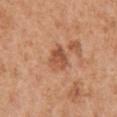Q: Was this lesion biopsied?
A: no biopsy performed (imaged during a skin exam)
Q: Where on the body is the lesion?
A: the arm
Q: Who is the patient?
A: female, aged around 40
Q: What is the lesion's diameter?
A: ~3 mm (longest diameter)
Q: How was the tile lit?
A: white-light
Q: What is the imaging modality?
A: ~15 mm crop, total-body skin-cancer survey